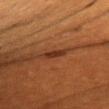biopsy status = catalogued during a skin exam; not biopsied
patient = female, roughly 55 years of age
location = the chest
imaging modality = ~15 mm tile from a whole-body skin photo